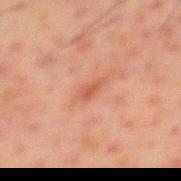| feature | finding |
|---|---|
| follow-up | no biopsy performed (imaged during a skin exam) |
| subject | male, about 50 years old |
| site | the mid back |
| size | about 2.5 mm |
| acquisition | ~15 mm tile from a whole-body skin photo |